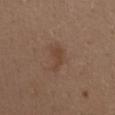Impression:
The lesion was tiled from a total-body skin photograph and was not biopsied.
Clinical summary:
Automated tile analysis of the lesion measured an average lesion color of about L≈43 a*≈18 b*≈27 (CIELAB) and roughly 6 lightness units darker than nearby skin. The analysis additionally found a color-variation rating of about 2/10 and radial color variation of about 1. And it measured an automated nevus-likeness rating near 10 out of 100. The tile uses white-light illumination. The patient is a female aged approximately 40. From the chest. Cropped from a whole-body photographic skin survey; the tile spans about 15 mm. Measured at roughly 3.5 mm in maximum diameter.